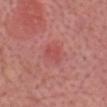follow-up: catalogued during a skin exam; not biopsied | image source: ~15 mm crop, total-body skin-cancer survey | subject: male, aged around 60 | body site: the chest.